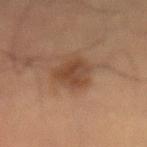Impression: Part of a total-body skin-imaging series; this lesion was reviewed on a skin check and was not flagged for biopsy. Clinical summary: Captured under cross-polarized illumination. The lesion is on the front of the torso. A 15 mm close-up tile from a total-body photography series done for melanoma screening. The recorded lesion diameter is about 4 mm. The lesion-visualizer software estimated a shape eccentricity near 0.5 and a shape-asymmetry score of about 0.3 (0 = symmetric). And it measured a border-irregularity index near 3/10, internal color variation of about 3.5 on a 0–10 scale, and a peripheral color-asymmetry measure near 1. The patient is a male aged approximately 55.